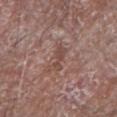Assessment: Recorded during total-body skin imaging; not selected for excision or biopsy. Acquisition and patient details: This image is a 15 mm lesion crop taken from a total-body photograph. This is a white-light tile. On the right forearm. A male patient, aged 78–82. The lesion's longest dimension is about 3.5 mm.